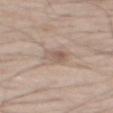Q: Was a biopsy performed?
A: total-body-photography surveillance lesion; no biopsy
Q: What is the anatomic site?
A: the mid back
Q: Who is the patient?
A: male, roughly 50 years of age
Q: How was the tile lit?
A: white-light
Q: What is the imaging modality?
A: total-body-photography crop, ~15 mm field of view